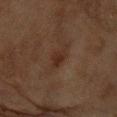Recorded during total-body skin imaging; not selected for excision or biopsy. Automated image analysis of the tile measured border irregularity of about 3 on a 0–10 scale, internal color variation of about 2 on a 0–10 scale, and peripheral color asymmetry of about 0.5. Cropped from a whole-body photographic skin survey; the tile spans about 15 mm. Captured under cross-polarized illumination. Located on the left forearm. A female subject, roughly 80 years of age. Approximately 2.5 mm at its widest.